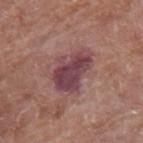No biopsy was performed on this lesion — it was imaged during a full skin examination and was not determined to be concerning. Approximately 5 mm at its widest. A region of skin cropped from a whole-body photographic capture, roughly 15 mm wide. The patient is a male aged 78–82. The lesion is on the left arm. The tile uses white-light illumination.